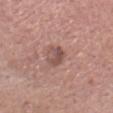The lesion was photographed on a routine skin check and not biopsied; there is no pathology result. A region of skin cropped from a whole-body photographic capture, roughly 15 mm wide. A male subject approximately 60 years of age. Located on the head or neck.